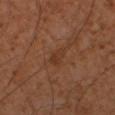Recorded during total-body skin imaging; not selected for excision or biopsy. This image is a 15 mm lesion crop taken from a total-body photograph. About 3 mm across. A male patient, aged 58 to 62. Captured under cross-polarized illumination. From the left upper arm.